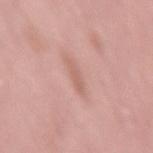Q: Was this lesion biopsied?
A: total-body-photography surveillance lesion; no biopsy
Q: Patient demographics?
A: male, aged 63 to 67
Q: How was this image acquired?
A: 15 mm crop, total-body photography
Q: Lesion location?
A: the right thigh